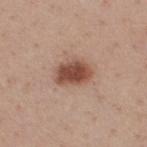This lesion was catalogued during total-body skin photography and was not selected for biopsy. A roughly 15 mm field-of-view crop from a total-body skin photograph. About 4 mm across. The lesion is on the upper back. Automated image analysis of the tile measured a lesion area of about 9 mm² and two-axis asymmetry of about 0.2. And it measured an average lesion color of about L≈49 a*≈22 b*≈28 (CIELAB), about 15 CIELAB-L* units darker than the surrounding skin, and a lesion-to-skin contrast of about 10.5 (normalized; higher = more distinct). And it measured a border-irregularity rating of about 2/10. The analysis additionally found an automated nevus-likeness rating near 100 out of 100 and a lesion-detection confidence of about 100/100. A male patient, approximately 40 years of age.